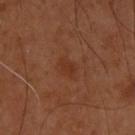follow-up — no biopsy performed (imaged during a skin exam) | patient — male, in their mid-50s | image — total-body-photography crop, ~15 mm field of view | anatomic site — the upper back.